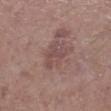follow-up = total-body-photography surveillance lesion; no biopsy.